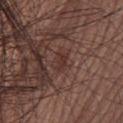notes: total-body-photography surveillance lesion; no biopsy
subject: female, in their mid-50s
tile lighting: white-light
anatomic site: the upper back
lesion diameter: about 2.5 mm
imaging modality: total-body-photography crop, ~15 mm field of view